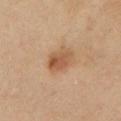This lesion was catalogued during total-body skin photography and was not selected for biopsy. A region of skin cropped from a whole-body photographic capture, roughly 15 mm wide. The recorded lesion diameter is about 3.5 mm. The total-body-photography lesion software estimated a border-irregularity rating of about 1.5/10 and a peripheral color-asymmetry measure near 1.5. It also reported a lesion-detection confidence of about 100/100. On the left upper arm. The subject is a female aged 58 to 62.